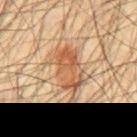Imaged during a routine full-body skin examination; the lesion was not biopsied and no histopathology is available. Measured at roughly 5.5 mm in maximum diameter. Captured under cross-polarized illumination. A male patient approximately 45 years of age. The lesion is located on the chest. A close-up tile cropped from a whole-body skin photograph, about 15 mm across.